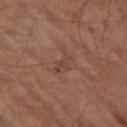Recorded during total-body skin imaging; not selected for excision or biopsy. On the left thigh. This image is a 15 mm lesion crop taken from a total-body photograph. The subject is a male about 65 years old. Automated tile analysis of the lesion measured an area of roughly 4.5 mm², an outline eccentricity of about 0.85 (0 = round, 1 = elongated), and a symmetry-axis asymmetry near 0.45. The analysis additionally found a mean CIELAB color near L≈40 a*≈17 b*≈25, about 5 CIELAB-L* units darker than the surrounding skin, and a normalized lesion–skin contrast near 4.5. The software also gave a nevus-likeness score of about 0/100 and a detector confidence of about 100 out of 100 that the crop contains a lesion.